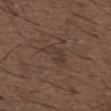• follow-up: total-body-photography surveillance lesion; no biopsy
• size: about 4.5 mm
• image-analysis metrics: internal color variation of about 2.5 on a 0–10 scale and peripheral color asymmetry of about 1; an automated nevus-likeness rating near 0 out of 100
• location: the upper back
• acquisition: ~15 mm crop, total-body skin-cancer survey
• subject: male, about 50 years old
• tile lighting: white-light